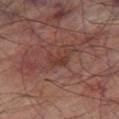| feature | finding |
|---|---|
| follow-up | no biopsy performed (imaged during a skin exam) |
| subject | male, aged approximately 75 |
| automated metrics | an area of roughly 5 mm², an eccentricity of roughly 0.7, and two-axis asymmetry of about 0.45; an average lesion color of about L≈31 a*≈19 b*≈21 (CIELAB) and a normalized lesion–skin contrast near 5.5; border irregularity of about 5.5 on a 0–10 scale, a color-variation rating of about 2/10, and peripheral color asymmetry of about 0.5 |
| location | the right thigh |
| lesion diameter | about 3 mm |
| tile lighting | cross-polarized |
| acquisition | total-body-photography crop, ~15 mm field of view |